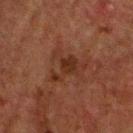<tbp_lesion>
  <patient>
    <sex>male</sex>
    <age_approx>60</age_approx>
  </patient>
  <image>
    <source>total-body photography crop</source>
    <field_of_view_mm>15</field_of_view_mm>
  </image>
  <site>head or neck</site>
  <lesion_size>
    <long_diameter_mm_approx>4.5</long_diameter_mm_approx>
  </lesion_size>
  <lighting>cross-polarized</lighting>
</tbp_lesion>